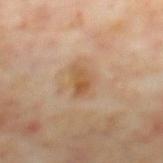Q: Is there a histopathology result?
A: total-body-photography surveillance lesion; no biopsy
Q: How was the tile lit?
A: cross-polarized illumination
Q: What kind of image is this?
A: 15 mm crop, total-body photography
Q: What is the anatomic site?
A: the mid back
Q: How large is the lesion?
A: ≈3 mm
Q: Automated lesion metrics?
A: a lesion area of about 5 mm² and an eccentricity of roughly 0.8; about 9 CIELAB-L* units darker than the surrounding skin; a border-irregularity index near 3.5/10; a nevus-likeness score of about 5/100 and a detector confidence of about 100 out of 100 that the crop contains a lesion
Q: What are the patient's age and sex?
A: male, aged approximately 70